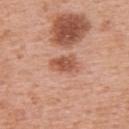{
  "biopsy_status": "not biopsied; imaged during a skin examination",
  "image": {
    "source": "total-body photography crop",
    "field_of_view_mm": 15
  },
  "site": "upper back",
  "lesion_size": {
    "long_diameter_mm_approx": 3.5
  },
  "patient": {
    "sex": "female",
    "age_approx": 50
  }
}